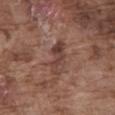Impression: No biopsy was performed on this lesion — it was imaged during a full skin examination and was not determined to be concerning. Clinical summary: The subject is a male in their mid- to late 70s. The lesion is on the abdomen. The tile uses white-light illumination. A 15 mm close-up extracted from a 3D total-body photography capture. The lesion-visualizer software estimated border irregularity of about 5 on a 0–10 scale, a within-lesion color-variation index near 3/10, and peripheral color asymmetry of about 0.5.